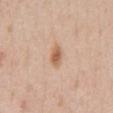Assessment: This lesion was catalogued during total-body skin photography and was not selected for biopsy. Acquisition and patient details: Cropped from a whole-body photographic skin survey; the tile spans about 15 mm. Longest diameter approximately 2.5 mm. On the chest. The subject is a male aged around 55. The tile uses white-light illumination.